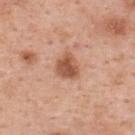Captured during whole-body skin photography for melanoma surveillance; the lesion was not biopsied. This image is a 15 mm lesion crop taken from a total-body photograph. A female subject, roughly 50 years of age. On the back. The lesion's longest dimension is about 3 mm. Captured under white-light illumination. An algorithmic analysis of the crop reported a border-irregularity index near 2/10, a color-variation rating of about 3.5/10, and peripheral color asymmetry of about 1. The software also gave lesion-presence confidence of about 100/100.